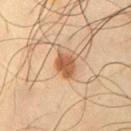site: the chest; size: ≈3 mm; patient: male, aged 63 to 67; image: 15 mm crop, total-body photography.